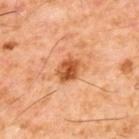No biopsy was performed on this lesion — it was imaged during a full skin examination and was not determined to be concerning.
The tile uses cross-polarized illumination.
Located on the upper back.
Automated tile analysis of the lesion measured a lesion area of about 7 mm², an outline eccentricity of about 0.6 (0 = round, 1 = elongated), and a shape-asymmetry score of about 0.2 (0 = symmetric). The software also gave a mean CIELAB color near L≈52 a*≈27 b*≈40 and a lesion–skin lightness drop of about 13. The software also gave border irregularity of about 2 on a 0–10 scale. It also reported a classifier nevus-likeness of about 85/100 and lesion-presence confidence of about 100/100.
A 15 mm close-up tile from a total-body photography series done for melanoma screening.
The subject is a male about 60 years old.